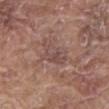The lesion was tiled from a total-body skin photograph and was not biopsied. A region of skin cropped from a whole-body photographic capture, roughly 15 mm wide. The patient is a male aged 78 to 82. Imaged with white-light lighting. From the mid back. The total-body-photography lesion software estimated a shape eccentricity near 0.85 and a shape-asymmetry score of about 0.35 (0 = symmetric). About 4.5 mm across.